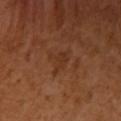Background:
On the right upper arm. A roughly 15 mm field-of-view crop from a total-body skin photograph. Automated image analysis of the tile measured a lesion color around L≈34 a*≈22 b*≈32 in CIELAB and roughly 5 lightness units darker than nearby skin. The software also gave a nevus-likeness score of about 0/100 and a detector confidence of about 100 out of 100 that the crop contains a lesion. A female subject, aged 53–57. Imaged with cross-polarized lighting.